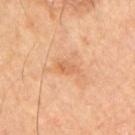follow-up: catalogued during a skin exam; not biopsied
TBP lesion metrics: a lesion area of about 3.5 mm² and an eccentricity of roughly 0.85; a classifier nevus-likeness of about 0/100 and a detector confidence of about 100 out of 100 that the crop contains a lesion
size: ~3 mm (longest diameter)
anatomic site: the right upper arm
patient: male, about 60 years old
acquisition: total-body-photography crop, ~15 mm field of view